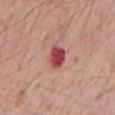A male subject aged 58 to 62.
Located on the mid back.
Captured under white-light illumination.
Automated image analysis of the tile measured a lesion color around L≈47 a*≈36 b*≈22 in CIELAB, roughly 17 lightness units darker than nearby skin, and a lesion-to-skin contrast of about 11.5 (normalized; higher = more distinct). The software also gave a border-irregularity rating of about 1.5/10, a color-variation rating of about 4/10, and a peripheral color-asymmetry measure near 1. And it measured a classifier nevus-likeness of about 0/100.
Cropped from a total-body skin-imaging series; the visible field is about 15 mm.